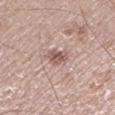image source = ~15 mm tile from a whole-body skin photo; body site = the leg; subject = male, aged 63 to 67; size = about 2.5 mm.